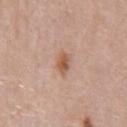Case summary:
- biopsy status · total-body-photography surveillance lesion; no biopsy